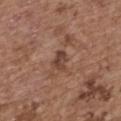Clinical impression: Captured during whole-body skin photography for melanoma surveillance; the lesion was not biopsied. Image and clinical context: This image is a 15 mm lesion crop taken from a total-body photograph. A female subject, roughly 65 years of age. On the upper back.